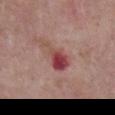Impression:
Part of a total-body skin-imaging series; this lesion was reviewed on a skin check and was not flagged for biopsy.
Image and clinical context:
The subject is a male in their mid- to late 60s. Located on the front of the torso. A close-up tile cropped from a whole-body skin photograph, about 15 mm across.